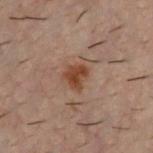Case summary:
* workup · total-body-photography surveillance lesion; no biopsy
* illumination · cross-polarized illumination
* body site · the front of the torso
* patient · male, aged 28 to 32
* image · ~15 mm crop, total-body skin-cancer survey
* size · about 3 mm